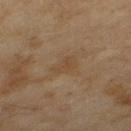Q: Was a biopsy performed?
A: total-body-photography surveillance lesion; no biopsy
Q: Patient demographics?
A: female, roughly 60 years of age
Q: What kind of image is this?
A: 15 mm crop, total-body photography
Q: Where on the body is the lesion?
A: the left thigh
Q: Automated lesion metrics?
A: a lesion area of about 3.5 mm², an outline eccentricity of about 0.8 (0 = round, 1 = elongated), and a symmetry-axis asymmetry near 0.4; border irregularity of about 3.5 on a 0–10 scale, a within-lesion color-variation index near 0.5/10, and a peripheral color-asymmetry measure near 0; an automated nevus-likeness rating near 0 out of 100 and a detector confidence of about 100 out of 100 that the crop contains a lesion
Q: What lighting was used for the tile?
A: cross-polarized illumination